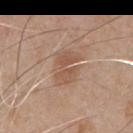biopsy status = imaged on a skin check; not biopsied
lighting = white-light
patient = male, aged 63 to 67
body site = the chest
lesion diameter = ≈4 mm
imaging modality = ~15 mm tile from a whole-body skin photo